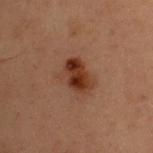<tbp_lesion>
  <biopsy_status>not biopsied; imaged during a skin examination</biopsy_status>
  <site>upper back</site>
  <patient>
    <sex>female</sex>
    <age_approx>40</age_approx>
  </patient>
  <image>
    <source>total-body photography crop</source>
    <field_of_view_mm>15</field_of_view_mm>
  </image>
</tbp_lesion>